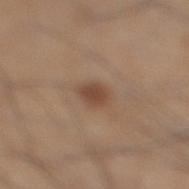The lesion was photographed on a routine skin check and not biopsied; there is no pathology result. This image is a 15 mm lesion crop taken from a total-body photograph. A male subject in their mid- to late 40s. About 2.5 mm across. Automated image analysis of the tile measured internal color variation of about 1.5 on a 0–10 scale and a peripheral color-asymmetry measure near 0.5. The analysis additionally found a classifier nevus-likeness of about 85/100 and a lesion-detection confidence of about 100/100. The lesion is located on the right lower leg.